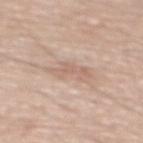Clinical impression:
The lesion was photographed on a routine skin check and not biopsied; there is no pathology result.
Context:
From the mid back. The tile uses white-light illumination. An algorithmic analysis of the crop reported a lesion color around L≈64 a*≈17 b*≈27 in CIELAB, a lesion–skin lightness drop of about 7, and a normalized lesion–skin contrast near 4.5. And it measured an automated nevus-likeness rating near 0 out of 100 and lesion-presence confidence of about 95/100. Longest diameter approximately 4.5 mm. A lesion tile, about 15 mm wide, cut from a 3D total-body photograph. A male patient aged approximately 70.